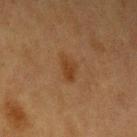biopsy_status: not biopsied; imaged during a skin examination
site: left upper arm
image:
  source: total-body photography crop
  field_of_view_mm: 15
patient:
  sex: female
  age_approx: 55
lighting: cross-polarized
lesion_size:
  long_diameter_mm_approx: 3.0